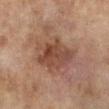notes: no biopsy performed (imaged during a skin exam); lesion diameter: ≈8.5 mm; patient: female, roughly 60 years of age; acquisition: ~15 mm crop, total-body skin-cancer survey; location: the leg; tile lighting: cross-polarized illumination.